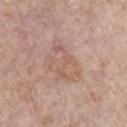notes=no biopsy performed (imaged during a skin exam) | size=about 4 mm | anatomic site=the front of the torso | illumination=white-light | patient=male, approximately 70 years of age | TBP lesion metrics=a lesion–skin lightness drop of about 7 and a normalized border contrast of about 5; a border-irregularity index near 10/10, a color-variation rating of about 0/10, and a peripheral color-asymmetry measure near 0; an automated nevus-likeness rating near 0 out of 100 and a lesion-detection confidence of about 100/100 | acquisition=15 mm crop, total-body photography.